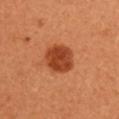A female subject aged 48–52.
A 15 mm crop from a total-body photograph taken for skin-cancer surveillance.
Measured at roughly 4 mm in maximum diameter.
Automated tile analysis of the lesion measured a shape eccentricity near 0.4 and two-axis asymmetry of about 0.1. It also reported a color-variation rating of about 3.5/10 and radial color variation of about 1.5. It also reported an automated nevus-likeness rating near 100 out of 100 and a lesion-detection confidence of about 100/100.
Imaged with cross-polarized lighting.